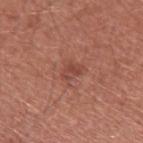Clinical impression: The lesion was tiled from a total-body skin photograph and was not biopsied. Context: Imaged with white-light lighting. On the left upper arm. A 15 mm close-up tile from a total-body photography series done for melanoma screening. A male patient aged 58–62. Measured at roughly 3 mm in maximum diameter.